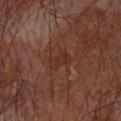{
  "biopsy_status": "not biopsied; imaged during a skin examination",
  "image": {
    "source": "total-body photography crop",
    "field_of_view_mm": 15
  },
  "patient": {
    "sex": "male",
    "age_approx": 65
  },
  "lesion_size": {
    "long_diameter_mm_approx": 2.5
  },
  "site": "arm"
}